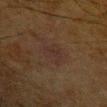  biopsy_status: not biopsied; imaged during a skin examination
  image:
    source: total-body photography crop
    field_of_view_mm: 15
  patient:
    sex: male
    age_approx: 65
  site: left upper arm